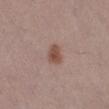Imaged during a routine full-body skin examination; the lesion was not biopsied and no histopathology is available. The total-body-photography lesion software estimated radial color variation of about 1. A female patient, aged approximately 30. Located on the right lower leg. This is a white-light tile. Cropped from a whole-body photographic skin survey; the tile spans about 15 mm.